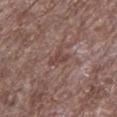Q: Was a biopsy performed?
A: imaged on a skin check; not biopsied
Q: Where on the body is the lesion?
A: the leg
Q: How was this image acquired?
A: total-body-photography crop, ~15 mm field of view
Q: Patient demographics?
A: male, approximately 70 years of age